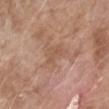Imaged during a routine full-body skin examination; the lesion was not biopsied and no histopathology is available. A female subject about 75 years old. Automated tile analysis of the lesion measured a lesion–skin lightness drop of about 6. The software also gave an automated nevus-likeness rating near 0 out of 100 and a lesion-detection confidence of about 100/100. On the right forearm. Imaged with white-light lighting. A 15 mm close-up extracted from a 3D total-body photography capture.